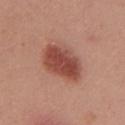This is a white-light tile. Cropped from a total-body skin-imaging series; the visible field is about 15 mm. About 6 mm across. The lesion is located on the mid back. A female patient, aged 23–27.